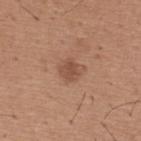Part of a total-body skin-imaging series; this lesion was reviewed on a skin check and was not flagged for biopsy.
The subject is a male aged 63–67.
Automated tile analysis of the lesion measured an area of roughly 5 mm² and an eccentricity of roughly 0.5. The analysis additionally found a lesion color around L≈50 a*≈22 b*≈29 in CIELAB. It also reported internal color variation of about 2 on a 0–10 scale and radial color variation of about 0.5.
Approximately 3 mm at its widest.
Captured under white-light illumination.
The lesion is located on the back.
Cropped from a whole-body photographic skin survey; the tile spans about 15 mm.